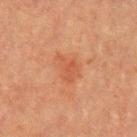tile lighting = cross-polarized
automated lesion analysis = a lesion color around L≈44 a*≈24 b*≈31 in CIELAB; a within-lesion color-variation index near 2/10 and a peripheral color-asymmetry measure near 0.5
lesion size = about 3.5 mm
subject = female, roughly 55 years of age
image = ~15 mm tile from a whole-body skin photo
site = the left upper arm